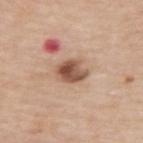Findings:
- biopsy status: total-body-photography surveillance lesion; no biopsy
- subject: male, about 65 years old
- size: ≈3.5 mm
- body site: the upper back
- lighting: white-light
- automated metrics: a lesion area of about 7 mm², an eccentricity of roughly 0.7, and two-axis asymmetry of about 0.2; a nevus-likeness score of about 0/100
- imaging modality: ~15 mm tile from a whole-body skin photo